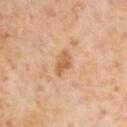Clinical impression:
Part of a total-body skin-imaging series; this lesion was reviewed on a skin check and was not flagged for biopsy.
Clinical summary:
The lesion is located on the left upper arm. Approximately 3 mm at its widest. A male patient, aged approximately 55. This image is a 15 mm lesion crop taken from a total-body photograph. Automated tile analysis of the lesion measured a lesion color around L≈60 a*≈22 b*≈40 in CIELAB, roughly 10 lightness units darker than nearby skin, and a normalized lesion–skin contrast near 7.5. The software also gave lesion-presence confidence of about 100/100. Imaged with cross-polarized lighting.